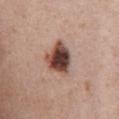Clinical impression:
No biopsy was performed on this lesion — it was imaged during a full skin examination and was not determined to be concerning.
Clinical summary:
On the front of the torso. A 15 mm close-up tile from a total-body photography series done for melanoma screening. The patient is a female aged 43 to 47. Automated image analysis of the tile measured a lesion area of about 12 mm², an eccentricity of roughly 0.65, and a shape-asymmetry score of about 0.25 (0 = symmetric). And it measured an automated nevus-likeness rating near 95 out of 100.